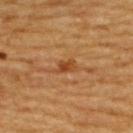<case>
<biopsy_status>not biopsied; imaged during a skin examination</biopsy_status>
<image>
  <source>total-body photography crop</source>
  <field_of_view_mm>15</field_of_view_mm>
</image>
<patient>
  <sex>male</sex>
  <age_approx>60</age_approx>
</patient>
<automated_metrics>
  <cielab_L>44</cielab_L>
  <cielab_a>25</cielab_a>
  <cielab_b>40</cielab_b>
  <vs_skin_darker_L>9.0</vs_skin_darker_L>
  <lesion_detection_confidence_0_100>100</lesion_detection_confidence_0_100>
</automated_metrics>
<lighting>cross-polarized</lighting>
<site>back</site>
</case>